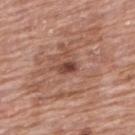Impression: Captured during whole-body skin photography for melanoma surveillance; the lesion was not biopsied. Image and clinical context: The total-body-photography lesion software estimated an outline eccentricity of about 0.8 (0 = round, 1 = elongated) and a symmetry-axis asymmetry near 0.25. The software also gave a border-irregularity index near 2/10, internal color variation of about 5 on a 0–10 scale, and a peripheral color-asymmetry measure near 2. The software also gave a classifier nevus-likeness of about 45/100. A roughly 15 mm field-of-view crop from a total-body skin photograph. The lesion is on the back. Captured under white-light illumination. Measured at roughly 2.5 mm in maximum diameter. A female subject, roughly 60 years of age.